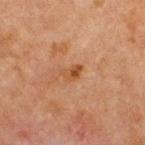Q: Is there a histopathology result?
A: no biopsy performed (imaged during a skin exam)
Q: How was this image acquired?
A: ~15 mm crop, total-body skin-cancer survey
Q: Automated lesion metrics?
A: a shape eccentricity near 0.8 and a shape-asymmetry score of about 0.45 (0 = symmetric); border irregularity of about 4 on a 0–10 scale and peripheral color asymmetry of about 1; an automated nevus-likeness rating near 20 out of 100
Q: Who is the patient?
A: male, in their mid-60s
Q: Illumination type?
A: cross-polarized
Q: Lesion location?
A: the chest